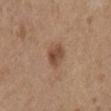workup: catalogued during a skin exam; not biopsied | subject: female, approximately 65 years of age | TBP lesion metrics: an average lesion color of about L≈47 a*≈19 b*≈29 (CIELAB), about 11 CIELAB-L* units darker than the surrounding skin, and a lesion-to-skin contrast of about 8 (normalized; higher = more distinct); a lesion-detection confidence of about 100/100 | site: the chest | lesion diameter: ≈3 mm | tile lighting: white-light | acquisition: ~15 mm tile from a whole-body skin photo.